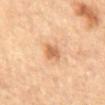Q: What lighting was used for the tile?
A: cross-polarized illumination
Q: What are the patient's age and sex?
A: male, in their mid- to late 80s or older
Q: Where on the body is the lesion?
A: the mid back
Q: How was this image acquired?
A: ~15 mm tile from a whole-body skin photo